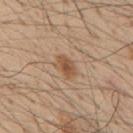Imaged during a routine full-body skin examination; the lesion was not biopsied and no histopathology is available. Cropped from a total-body skin-imaging series; the visible field is about 15 mm. The lesion is located on the mid back. Imaged with white-light lighting. The total-body-photography lesion software estimated a border-irregularity index near 2.5/10 and a peripheral color-asymmetry measure near 0.5. The software also gave a classifier nevus-likeness of about 70/100. A male subject, aged approximately 55.